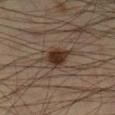Impression:
The lesion was photographed on a routine skin check and not biopsied; there is no pathology result.
Acquisition and patient details:
On the left lower leg. About 4.5 mm across. A male subject, aged 33–37. A roughly 15 mm field-of-view crop from a total-body skin photograph.